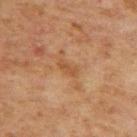Captured during whole-body skin photography for melanoma surveillance; the lesion was not biopsied. The lesion is on the upper back. A female subject, roughly 60 years of age. A 15 mm close-up tile from a total-body photography series done for melanoma screening. Captured under cross-polarized illumination. The total-body-photography lesion software estimated a lesion–skin lightness drop of about 6 and a normalized lesion–skin contrast near 5.5. It also reported border irregularity of about 2.5 on a 0–10 scale and a color-variation rating of about 0/10.